This is a white-light tile; the subject is a male roughly 60 years of age; the recorded lesion diameter is about 1 mm; the lesion is on the head or neck; The total-body-photography lesion software estimated a lesion-to-skin contrast of about 5.5 (normalized; higher = more distinct). The software also gave a border-irregularity rating of about 3.5/10 and a peripheral color-asymmetry measure near 0. And it measured an automated nevus-likeness rating near 0 out of 100 and a detector confidence of about 0 out of 100 that the crop contains a lesion; a lesion tile, about 15 mm wide, cut from a 3D total-body photograph: 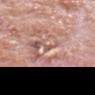On biopsy, histopathology showed an actinic keratosis, classified as an indeterminate (borderline) lesion.